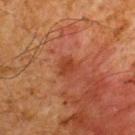This lesion was catalogued during total-body skin photography and was not selected for biopsy.
A male patient, aged around 60.
The tile uses cross-polarized illumination.
About 2.5 mm across.
The lesion is on the upper back.
A region of skin cropped from a whole-body photographic capture, roughly 15 mm wide.